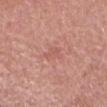Clinical impression: Recorded during total-body skin imaging; not selected for excision or biopsy. Context: Imaged with white-light lighting. The lesion is located on the head or neck. A male subject approximately 60 years of age. Cropped from a total-body skin-imaging series; the visible field is about 15 mm. The lesion's longest dimension is about 2.5 mm.